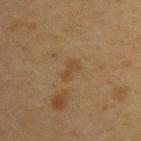Clinical impression: The lesion was photographed on a routine skin check and not biopsied; there is no pathology result. Acquisition and patient details: A female patient aged 38 to 42. Located on the upper back. Approximately 2.5 mm at its widest. This is a cross-polarized tile. This image is a 15 mm lesion crop taken from a total-body photograph.